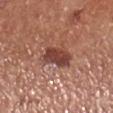Findings:
* patient: male, in their 70s
* illumination: white-light illumination
* site: the leg
* automated metrics: a lesion area of about 8.5 mm²; a border-irregularity rating of about 2.5/10; lesion-presence confidence of about 100/100
* size: ~4.5 mm (longest diameter)
* image: ~15 mm crop, total-body skin-cancer survey